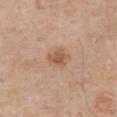No biopsy was performed on this lesion — it was imaged during a full skin examination and was not determined to be concerning.
The lesion is on the chest.
The tile uses white-light illumination.
A male subject, aged approximately 80.
The recorded lesion diameter is about 3 mm.
An algorithmic analysis of the crop reported border irregularity of about 2 on a 0–10 scale, internal color variation of about 2 on a 0–10 scale, and peripheral color asymmetry of about 0.5.
A 15 mm close-up tile from a total-body photography series done for melanoma screening.